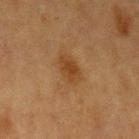Assessment:
Imaged during a routine full-body skin examination; the lesion was not biopsied and no histopathology is available.
Context:
A 15 mm crop from a total-body photograph taken for skin-cancer surveillance. The lesion is on the arm. The subject is a female roughly 55 years of age.